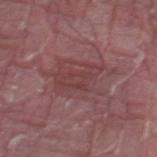Clinical impression:
Part of a total-body skin-imaging series; this lesion was reviewed on a skin check and was not flagged for biopsy.
Context:
The tile uses white-light illumination. The subject is a male aged 38–42. Automated image analysis of the tile measured an area of roughly 18 mm², an outline eccentricity of about 0.75 (0 = round, 1 = elongated), and a symmetry-axis asymmetry near 0.3. It also reported border irregularity of about 6 on a 0–10 scale. Cropped from a whole-body photographic skin survey; the tile spans about 15 mm. From the right thigh. The lesion's longest dimension is about 7 mm.